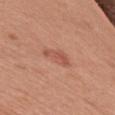This lesion was catalogued during total-body skin photography and was not selected for biopsy. An algorithmic analysis of the crop reported an area of roughly 5.5 mm² and an outline eccentricity of about 0.85 (0 = round, 1 = elongated). The software also gave a mean CIELAB color near L≈54 a*≈26 b*≈30, a lesion–skin lightness drop of about 8, and a normalized lesion–skin contrast near 5.5. It also reported a classifier nevus-likeness of about 15/100 and a detector confidence of about 100 out of 100 that the crop contains a lesion. Located on the right upper arm. Approximately 3.5 mm at its widest. A lesion tile, about 15 mm wide, cut from a 3D total-body photograph. This is a white-light tile. A male subject, aged around 70.